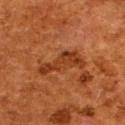Q: Was a biopsy performed?
A: imaged on a skin check; not biopsied
Q: What kind of image is this?
A: ~15 mm tile from a whole-body skin photo
Q: Illumination type?
A: cross-polarized
Q: Where on the body is the lesion?
A: the back
Q: Automated lesion metrics?
A: a border-irregularity rating of about 6/10 and a within-lesion color-variation index near 2.5/10
Q: Patient demographics?
A: female, in their 50s
Q: What is the lesion's diameter?
A: about 6 mm